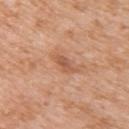The lesion was tiled from a total-body skin photograph and was not biopsied.